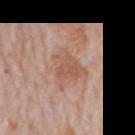No biopsy was performed on this lesion — it was imaged during a full skin examination and was not determined to be concerning. A roughly 15 mm field-of-view crop from a total-body skin photograph. A male patient aged around 80. From the mid back.